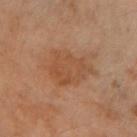A female subject aged around 60. The lesion is on the left arm. A lesion tile, about 15 mm wide, cut from a 3D total-body photograph.